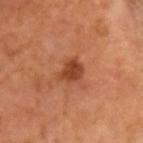Recorded during total-body skin imaging; not selected for excision or biopsy. A male patient, aged 63 to 67. A close-up tile cropped from a whole-body skin photograph, about 15 mm across. The tile uses cross-polarized illumination. Approximately 3 mm at its widest. Automated tile analysis of the lesion measured a lesion area of about 5.5 mm², an outline eccentricity of about 0.7 (0 = round, 1 = elongated), and two-axis asymmetry of about 0.3. It also reported an average lesion color of about L≈42 a*≈28 b*≈36 (CIELAB), roughly 11 lightness units darker than nearby skin, and a normalized border contrast of about 9. And it measured a nevus-likeness score of about 75/100 and a lesion-detection confidence of about 100/100.